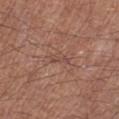Case summary:
* biopsy status — imaged on a skin check; not biopsied
* acquisition — total-body-photography crop, ~15 mm field of view
* subject — male, in their 70s
* location — the left lower leg